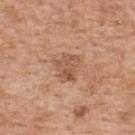follow-up — imaged on a skin check; not biopsied | site — the upper back | acquisition — ~15 mm crop, total-body skin-cancer survey | diameter — ≈3.5 mm | subject — male, approximately 60 years of age.